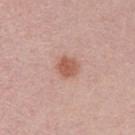The lesion was tiled from a total-body skin photograph and was not biopsied.
A male patient approximately 30 years of age.
Automated image analysis of the tile measured a lesion area of about 5.5 mm², an eccentricity of roughly 0.45, and two-axis asymmetry of about 0.2. It also reported a mean CIELAB color near L≈57 a*≈23 b*≈29, a lesion–skin lightness drop of about 10, and a lesion-to-skin contrast of about 8 (normalized; higher = more distinct).
Measured at roughly 2.5 mm in maximum diameter.
Imaged with white-light lighting.
Cropped from a total-body skin-imaging series; the visible field is about 15 mm.